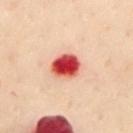Assessment: Part of a total-body skin-imaging series; this lesion was reviewed on a skin check and was not flagged for biopsy. Image and clinical context: The patient is a female about 60 years old. This is a cross-polarized tile. The lesion-visualizer software estimated a footprint of about 8.5 mm², an eccentricity of roughly 0.55, and a shape-asymmetry score of about 0.1 (0 = symmetric). The software also gave an average lesion color of about L≈47 a*≈38 b*≈30 (CIELAB), roughly 21 lightness units darker than nearby skin, and a lesion-to-skin contrast of about 14.5 (normalized; higher = more distinct). It also reported a nevus-likeness score of about 0/100. Approximately 3.5 mm at its widest. Cropped from a whole-body photographic skin survey; the tile spans about 15 mm. The lesion is located on the chest.